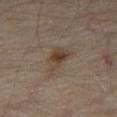Q: Was this lesion biopsied?
A: imaged on a skin check; not biopsied
Q: Who is the patient?
A: about 55 years old
Q: Automated lesion metrics?
A: a footprint of about 7 mm² and an eccentricity of roughly 0.75; a border-irregularity index near 4.5/10, internal color variation of about 5.5 on a 0–10 scale, and a peripheral color-asymmetry measure near 2
Q: Illumination type?
A: cross-polarized
Q: What is the imaging modality?
A: 15 mm crop, total-body photography
Q: Lesion size?
A: ~4 mm (longest diameter)
Q: Where on the body is the lesion?
A: the right thigh